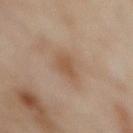Case summary:
- workup · total-body-photography surveillance lesion; no biopsy
- subject · female, aged 48–52
- image · total-body-photography crop, ~15 mm field of view
- illumination · cross-polarized
- site · the abdomen